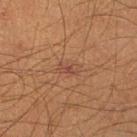Background: About 2.5 mm across. A male subject aged around 65. Captured under cross-polarized illumination. Cropped from a total-body skin-imaging series; the visible field is about 15 mm. The lesion is on the right lower leg.